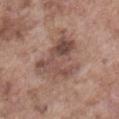Findings:
• workup: catalogued during a skin exam; not biopsied
• automated metrics: an area of roughly 21 mm² and a shape eccentricity near 0.7; border irregularity of about 7 on a 0–10 scale and peripheral color asymmetry of about 2.5; a classifier nevus-likeness of about 5/100 and a detector confidence of about 100 out of 100 that the crop contains a lesion
• acquisition: ~15 mm tile from a whole-body skin photo
• illumination: white-light illumination
• site: the abdomen
• subject: male, about 75 years old
• size: ≈6.5 mm